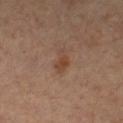follow-up: catalogued during a skin exam; not biopsied
lighting: cross-polarized illumination
anatomic site: the chest
subject: female, in their mid-40s
automated metrics: an outline eccentricity of about 0.8 (0 = round, 1 = elongated)
imaging modality: total-body-photography crop, ~15 mm field of view